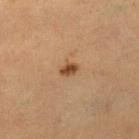Assessment: No biopsy was performed on this lesion — it was imaged during a full skin examination and was not determined to be concerning. Image and clinical context: A female subject aged 38 to 42. Cropped from a whole-body photographic skin survey; the tile spans about 15 mm. From the right lower leg.